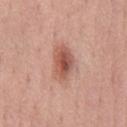The subject is a male about 55 years old.
Captured under white-light illumination.
A 15 mm close-up extracted from a 3D total-body photography capture.
From the chest.